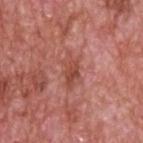The lesion was tiled from a total-body skin photograph and was not biopsied. The recorded lesion diameter is about 4.5 mm. Cropped from a total-body skin-imaging series; the visible field is about 15 mm. A male subject about 60 years old. The lesion is on the upper back. This is a white-light tile.